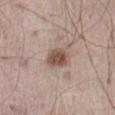Clinical impression: The lesion was tiled from a total-body skin photograph and was not biopsied. Acquisition and patient details: The lesion is on the abdomen. A male patient roughly 65 years of age. A close-up tile cropped from a whole-body skin photograph, about 15 mm across. Captured under white-light illumination. Measured at roughly 3 mm in maximum diameter.